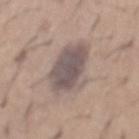Clinical impression:
Imaged during a routine full-body skin examination; the lesion was not biopsied and no histopathology is available.